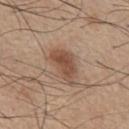- biopsy status: imaged on a skin check; not biopsied
- anatomic site: the chest
- patient: male, aged 53 to 57
- diameter: ≈4.5 mm
- acquisition: ~15 mm crop, total-body skin-cancer survey